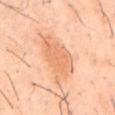Clinical impression: No biopsy was performed on this lesion — it was imaged during a full skin examination and was not determined to be concerning. Acquisition and patient details: A male patient, approximately 40 years of age. A lesion tile, about 15 mm wide, cut from a 3D total-body photograph. Located on the mid back.